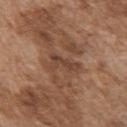biopsy status — catalogued during a skin exam; not biopsied | acquisition — ~15 mm crop, total-body skin-cancer survey | site — the chest | tile lighting — white-light illumination | diameter — ~3.5 mm (longest diameter) | patient — male, about 75 years old.